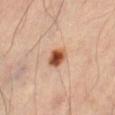{
  "biopsy_status": "not biopsied; imaged during a skin examination"
}